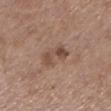biopsy status = catalogued during a skin exam; not biopsied | imaging modality = ~15 mm tile from a whole-body skin photo | anatomic site = the left lower leg | patient = female, roughly 65 years of age.